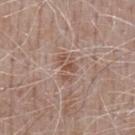Case summary:
• follow-up — no biopsy performed (imaged during a skin exam)
• site — the front of the torso
• diameter — about 3.5 mm
• image — ~15 mm crop, total-body skin-cancer survey
• subject — male, in their mid-70s